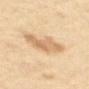Recorded during total-body skin imaging; not selected for excision or biopsy. Longest diameter approximately 5.5 mm. The lesion is on the upper back. A female subject, aged approximately 60. Captured under cross-polarized illumination. A roughly 15 mm field-of-view crop from a total-body skin photograph.